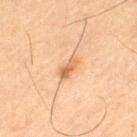No biopsy was performed on this lesion — it was imaged during a full skin examination and was not determined to be concerning. Cropped from a whole-body photographic skin survey; the tile spans about 15 mm. A male subject, in their mid-60s. The lesion is located on the left thigh. The recorded lesion diameter is about 2.5 mm.